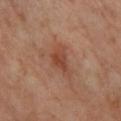Captured during whole-body skin photography for melanoma surveillance; the lesion was not biopsied. On the right lower leg. A female patient, roughly 55 years of age. Captured under cross-polarized illumination. The lesion-visualizer software estimated internal color variation of about 3 on a 0–10 scale. And it measured a nevus-likeness score of about 25/100 and a lesion-detection confidence of about 100/100. A roughly 15 mm field-of-view crop from a total-body skin photograph. Longest diameter approximately 4 mm.